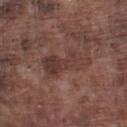No biopsy was performed on this lesion — it was imaged during a full skin examination and was not determined to be concerning.
On the left lower leg.
The subject is a male approximately 75 years of age.
The lesion's longest dimension is about 6 mm.
A close-up tile cropped from a whole-body skin photograph, about 15 mm across.